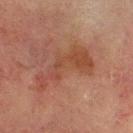| key | value |
|---|---|
| workup | catalogued during a skin exam; not biopsied |
| image source | ~15 mm crop, total-body skin-cancer survey |
| illumination | cross-polarized illumination |
| anatomic site | the chest |
| size | ≈7 mm |
| subject | male, aged around 70 |
| TBP lesion metrics | a footprint of about 15 mm²; a border-irregularity index near 8/10, internal color variation of about 3 on a 0–10 scale, and a peripheral color-asymmetry measure near 1; a nevus-likeness score of about 0/100 and lesion-presence confidence of about 100/100 |